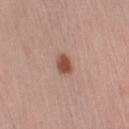Part of a total-body skin-imaging series; this lesion was reviewed on a skin check and was not flagged for biopsy. A male subject, about 55 years old. This is a white-light tile. The total-body-photography lesion software estimated a lesion area of about 4.5 mm² and a shape eccentricity near 0.65. It also reported an automated nevus-likeness rating near 100 out of 100 and a lesion-detection confidence of about 100/100. A roughly 15 mm field-of-view crop from a total-body skin photograph. The lesion is located on the left upper arm.